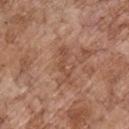Recorded during total-body skin imaging; not selected for excision or biopsy.
Automated image analysis of the tile measured a mean CIELAB color near L≈49 a*≈22 b*≈30, about 8 CIELAB-L* units darker than the surrounding skin, and a normalized lesion–skin contrast near 6. It also reported border irregularity of about 8.5 on a 0–10 scale, a color-variation rating of about 0/10, and a peripheral color-asymmetry measure near 0. It also reported a lesion-detection confidence of about 100/100.
This image is a 15 mm lesion crop taken from a total-body photograph.
From the chest.
A male patient, aged 68–72.
The recorded lesion diameter is about 4.5 mm.
Imaged with white-light lighting.